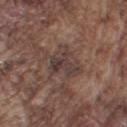follow-up: no biopsy performed (imaged during a skin exam) | subject: male, approximately 75 years of age | tile lighting: white-light | site: the left upper arm | lesion diameter: ~4.5 mm (longest diameter) | acquisition: 15 mm crop, total-body photography.